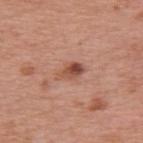| key | value |
|---|---|
| biopsy status | no biopsy performed (imaged during a skin exam) |
| acquisition | 15 mm crop, total-body photography |
| subject | female, roughly 40 years of age |
| location | the back |